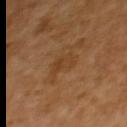notes: imaged on a skin check; not biopsied | image: ~15 mm crop, total-body skin-cancer survey | site: the upper back | patient: female, aged around 65 | illumination: cross-polarized.